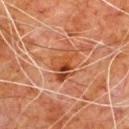biopsy_status: not biopsied; imaged during a skin examination
patient:
  sex: male
  age_approx: 80
site: chest
image:
  source: total-body photography crop
  field_of_view_mm: 15
lesion_size:
  long_diameter_mm_approx: 5.0